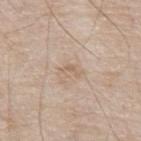Image and clinical context:
A roughly 15 mm field-of-view crop from a total-body skin photograph. The lesion is on the abdomen. A male patient, about 80 years old.